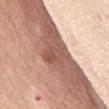| key | value |
|---|---|
| biopsy status | catalogued during a skin exam; not biopsied |
| lighting | white-light illumination |
| acquisition | ~15 mm crop, total-body skin-cancer survey |
| patient | female, roughly 55 years of age |
| size | about 3 mm |
| location | the chest |